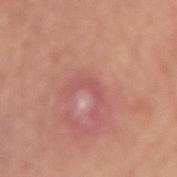<lesion>
<image>
  <source>total-body photography crop</source>
  <field_of_view_mm>15</field_of_view_mm>
</image>
<lighting>white-light</lighting>
<patient>
  <sex>male</sex>
  <age_approx>70</age_approx>
</patient>
<site>right upper arm</site>
<lesion_size>
  <long_diameter_mm_approx>3.0</long_diameter_mm_approx>
</lesion_size>
<diagnosis>
  <histopathology>verruca</histopathology>
  <malignancy>benign</malignancy>
  <taxonomic_path>Benign, Inflammatory or infectious diseases, Verruca</taxonomic_path>
</diagnosis>
</lesion>